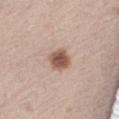| feature | finding |
|---|---|
| follow-up | total-body-photography surveillance lesion; no biopsy |
| imaging modality | 15 mm crop, total-body photography |
| lesion size | ~3 mm (longest diameter) |
| location | the abdomen |
| illumination | white-light illumination |
| patient | male, aged approximately 65 |